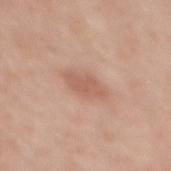<tbp_lesion>
  <biopsy_status>not biopsied; imaged during a skin examination</biopsy_status>
  <automated_metrics>
    <area_mm2_approx>7.0</area_mm2_approx>
    <eccentricity>0.85</eccentricity>
    <cielab_L>59</cielab_L>
    <cielab_a>22</cielab_a>
    <cielab_b>29</cielab_b>
    <vs_skin_darker_L>8.0</vs_skin_darker_L>
    <vs_skin_contrast_norm>5.5</vs_skin_contrast_norm>
    <peripheral_color_asymmetry>0.5</peripheral_color_asymmetry>
  </automated_metrics>
  <site>mid back</site>
  <lighting>white-light</lighting>
  <lesion_size>
    <long_diameter_mm_approx>4.5</long_diameter_mm_approx>
  </lesion_size>
  <patient>
    <sex>female</sex>
    <age_approx>35</age_approx>
  </patient>
  <image>
    <source>total-body photography crop</source>
    <field_of_view_mm>15</field_of_view_mm>
  </image>
</tbp_lesion>